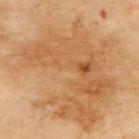Part of a total-body skin-imaging series; this lesion was reviewed on a skin check and was not flagged for biopsy. Automated image analysis of the tile measured a normalized lesion–skin contrast near 5.5. It also reported radial color variation of about 2. Approximately 15.5 mm at its widest. Imaged with cross-polarized lighting. On the upper back. A female subject aged 53 to 57. A 15 mm crop from a total-body photograph taken for skin-cancer surveillance.